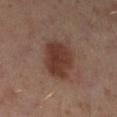Assessment: The lesion was tiled from a total-body skin photograph and was not biopsied. Acquisition and patient details: The lesion's longest dimension is about 5 mm. On the left lower leg. The lesion-visualizer software estimated a footprint of about 17 mm² and two-axis asymmetry of about 0.15. And it measured roughly 10 lightness units darker than nearby skin and a normalized lesion–skin contrast near 8.5. Cropped from a whole-body photographic skin survey; the tile spans about 15 mm. Imaged with cross-polarized lighting. A female patient, aged around 40.